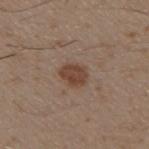{
  "biopsy_status": "not biopsied; imaged during a skin examination"
}